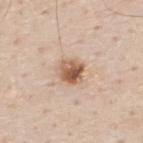Captured during whole-body skin photography for melanoma surveillance; the lesion was not biopsied.